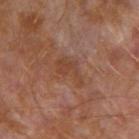Imaged during a routine full-body skin examination; the lesion was not biopsied and no histopathology is available.
The lesion's longest dimension is about 4 mm.
A roughly 15 mm field-of-view crop from a total-body skin photograph.
From the left arm.
Captured under cross-polarized illumination.
A male subject about 30 years old.